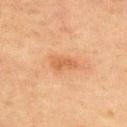Clinical impression:
Captured during whole-body skin photography for melanoma surveillance; the lesion was not biopsied.
Background:
A male patient aged 63 to 67. A roughly 15 mm field-of-view crop from a total-body skin photograph. On the chest. Approximately 3 mm at its widest. Imaged with cross-polarized lighting.